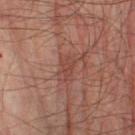Q: Was a biopsy performed?
A: total-body-photography surveillance lesion; no biopsy
Q: Who is the patient?
A: male, aged around 75
Q: What is the anatomic site?
A: the right thigh
Q: How was the tile lit?
A: cross-polarized illumination
Q: What is the imaging modality?
A: ~15 mm crop, total-body skin-cancer survey
Q: What did automated image analysis measure?
A: about 6 CIELAB-L* units darker than the surrounding skin and a normalized lesion–skin contrast near 5
Q: What is the lesion's diameter?
A: ~3 mm (longest diameter)